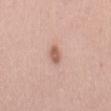notes: no biopsy performed (imaged during a skin exam) | lesion size: about 2.5 mm | image-analysis metrics: a mean CIELAB color near L≈60 a*≈21 b*≈28, a lesion–skin lightness drop of about 12, and a normalized border contrast of about 8; a border-irregularity index near 2.5/10 and a within-lesion color-variation index near 2/10; lesion-presence confidence of about 100/100 | acquisition: ~15 mm tile from a whole-body skin photo | location: the mid back | illumination: white-light illumination | subject: female, aged 58 to 62.